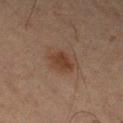Captured during whole-body skin photography for melanoma surveillance; the lesion was not biopsied. The recorded lesion diameter is about 3 mm. A male subject in their mid-60s. On the left lower leg. Imaged with cross-polarized lighting. A close-up tile cropped from a whole-body skin photograph, about 15 mm across.